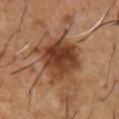Case summary:
• notes · catalogued during a skin exam; not biopsied
• patient · male, in their mid- to late 50s
• site · the front of the torso
• automated metrics · an area of roughly 23 mm² and a shape-asymmetry score of about 0.25 (0 = symmetric); a lesion-to-skin contrast of about 10 (normalized; higher = more distinct); a nevus-likeness score of about 85/100 and a detector confidence of about 100 out of 100 that the crop contains a lesion
• acquisition · 15 mm crop, total-body photography
• size · ~6 mm (longest diameter)
• lighting · cross-polarized illumination